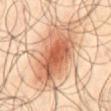Recorded during total-body skin imaging; not selected for excision or biopsy. On the abdomen. A 15 mm crop from a total-body photograph taken for skin-cancer surveillance. The tile uses cross-polarized illumination. The subject is a male in their mid-60s. Longest diameter approximately 9 mm.